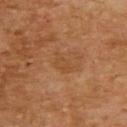biopsy status=catalogued during a skin exam; not biopsied.